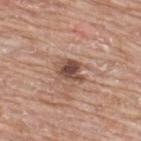{"biopsy_status": "not biopsied; imaged during a skin examination", "site": "upper back", "lighting": "white-light", "patient": {"sex": "male", "age_approx": 65}, "image": {"source": "total-body photography crop", "field_of_view_mm": 15}}